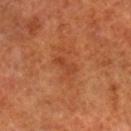Notes:
- notes: catalogued during a skin exam; not biopsied